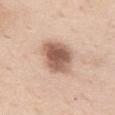* follow-up: total-body-photography surveillance lesion; no biopsy
* patient: female, about 55 years old
* acquisition: 15 mm crop, total-body photography
* location: the left thigh